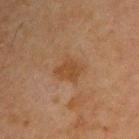Acquisition and patient details: Imaged with cross-polarized lighting. A 15 mm crop from a total-body photograph taken for skin-cancer surveillance. The patient is a male approximately 45 years of age. The lesion is located on the right upper arm. Approximately 3 mm at its widest.